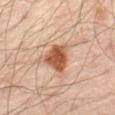image source=15 mm crop, total-body photography; patient=male, about 70 years old; location=the abdomen.